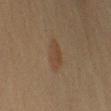biopsy_status: not biopsied; imaged during a skin examination
site: arm
lighting: cross-polarized
lesion_size:
  long_diameter_mm_approx: 4.0
patient:
  sex: female
  age_approx: 50
image:
  source: total-body photography crop
  field_of_view_mm: 15